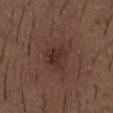Clinical impression: Captured during whole-body skin photography for melanoma surveillance; the lesion was not biopsied. Image and clinical context: A lesion tile, about 15 mm wide, cut from a 3D total-body photograph. A male subject, approximately 50 years of age. Captured under white-light illumination. The lesion-visualizer software estimated an outline eccentricity of about 0.5 (0 = round, 1 = elongated) and a symmetry-axis asymmetry near 0.4. The software also gave a mean CIELAB color near L≈31 a*≈19 b*≈21, about 7 CIELAB-L* units darker than the surrounding skin, and a normalized lesion–skin contrast near 7. And it measured a border-irregularity index near 4/10 and a within-lesion color-variation index near 3.5/10. The software also gave a nevus-likeness score of about 30/100 and a lesion-detection confidence of about 100/100. The lesion's longest dimension is about 3.5 mm. The lesion is on the chest.